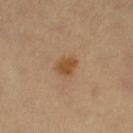  biopsy_status: not biopsied; imaged during a skin examination
  site: right thigh
  patient:
    sex: female
    age_approx: 70
  image:
    source: total-body photography crop
    field_of_view_mm: 15